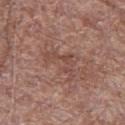This is a white-light tile.
The lesion's longest dimension is about 4.5 mm.
A close-up tile cropped from a whole-body skin photograph, about 15 mm across.
The patient is a male aged approximately 70.
On the leg.